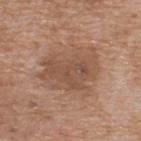No biopsy was performed on this lesion — it was imaged during a full skin examination and was not determined to be concerning. Measured at roughly 6.5 mm in maximum diameter. The subject is a male about 60 years old. The lesion is located on the back. The lesion-visualizer software estimated an area of roughly 26 mm² and two-axis asymmetry of about 0.3. It also reported border irregularity of about 4 on a 0–10 scale, a color-variation rating of about 4/10, and peripheral color asymmetry of about 1.5. The analysis additionally found lesion-presence confidence of about 100/100. This is a white-light tile. A close-up tile cropped from a whole-body skin photograph, about 15 mm across.